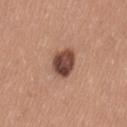The lesion is on the left thigh. The tile uses white-light illumination. The subject is a female aged 33 to 37. The lesion's longest dimension is about 3.5 mm. A lesion tile, about 15 mm wide, cut from a 3D total-body photograph.